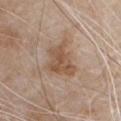Captured during whole-body skin photography for melanoma surveillance; the lesion was not biopsied.
A 15 mm close-up extracted from a 3D total-body photography capture.
The lesion-visualizer software estimated a footprint of about 12 mm², a shape eccentricity near 0.65, and a symmetry-axis asymmetry near 0.3. And it measured a border-irregularity index near 4/10 and internal color variation of about 3.5 on a 0–10 scale.
From the chest.
Captured under white-light illumination.
About 5 mm across.
A male subject, in their 80s.